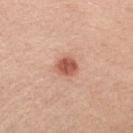Part of a total-body skin-imaging series; this lesion was reviewed on a skin check and was not flagged for biopsy.
The lesion is on the upper back.
A 15 mm close-up extracted from a 3D total-body photography capture.
A male subject aged approximately 60.